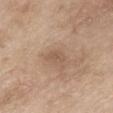Q: Lesion size?
A: about 2.5 mm
Q: What is the imaging modality?
A: ~15 mm tile from a whole-body skin photo
Q: What is the anatomic site?
A: the mid back
Q: Illumination type?
A: white-light
Q: What did automated image analysis measure?
A: a border-irregularity rating of about 2.5/10 and a color-variation rating of about 0/10
Q: Who is the patient?
A: female, aged 73 to 77